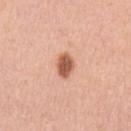Impression: No biopsy was performed on this lesion — it was imaged during a full skin examination and was not determined to be concerning. Background: The subject is a female aged 43–47. The lesion is located on the left upper arm. This image is a 15 mm lesion crop taken from a total-body photograph. Imaged with white-light lighting.